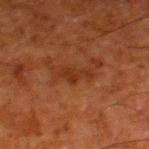Findings:
• follow-up · total-body-photography surveillance lesion; no biopsy
• acquisition · 15 mm crop, total-body photography
• subject · male, aged approximately 80
• site · the leg